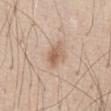Clinical impression:
No biopsy was performed on this lesion — it was imaged during a full skin examination and was not determined to be concerning.
Clinical summary:
A close-up tile cropped from a whole-body skin photograph, about 15 mm across. Automated tile analysis of the lesion measured a lesion color around L≈61 a*≈17 b*≈30 in CIELAB, roughly 10 lightness units darker than nearby skin, and a normalized border contrast of about 7. It also reported a border-irregularity index near 2.5/10 and peripheral color asymmetry of about 1.5. The analysis additionally found lesion-presence confidence of about 100/100. The tile uses white-light illumination. The lesion is on the abdomen. A male subject aged 43–47. Measured at roughly 3 mm in maximum diameter.